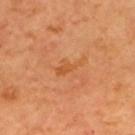Impression: Captured during whole-body skin photography for melanoma surveillance; the lesion was not biopsied. Image and clinical context: Approximately 3.5 mm at its widest. On the back. A lesion tile, about 15 mm wide, cut from a 3D total-body photograph. This is a cross-polarized tile. A male patient in their mid- to late 60s. Automated tile analysis of the lesion measured a footprint of about 4 mm², an eccentricity of roughly 0.9, and two-axis asymmetry of about 0.45. And it measured border irregularity of about 5.5 on a 0–10 scale, a within-lesion color-variation index near 1/10, and a peripheral color-asymmetry measure near 0.